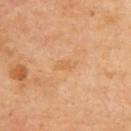Q: Is there a histopathology result?
A: no biopsy performed (imaged during a skin exam)
Q: How was this image acquired?
A: total-body-photography crop, ~15 mm field of view
Q: Lesion location?
A: the upper back
Q: Who is the patient?
A: male, in their 70s